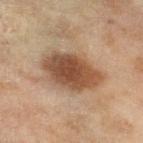biopsy_status: not biopsied; imaged during a skin examination
patient:
  sex: female
  age_approx: 60
lighting: cross-polarized
lesion_size:
  long_diameter_mm_approx: 7.0
automated_metrics:
  area_mm2_approx: 21.0
  eccentricity: 0.8
  cielab_L: 45
  cielab_a: 18
  cielab_b: 29
  border_irregularity_0_10: 2.0
  color_variation_0_10: 5.0
  peripheral_color_asymmetry: 1.5
image:
  source: total-body photography crop
  field_of_view_mm: 15
site: leg